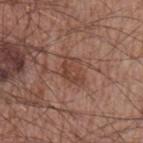Clinical impression: The lesion was photographed on a routine skin check and not biopsied; there is no pathology result. Clinical summary: A male subject aged 63 to 67. The lesion's longest dimension is about 3.5 mm. A 15 mm close-up tile from a total-body photography series done for melanoma screening. Located on the left upper arm. This is a white-light tile. The total-body-photography lesion software estimated an average lesion color of about L≈43 a*≈21 b*≈27 (CIELAB) and a normalized border contrast of about 6.5. The analysis additionally found a within-lesion color-variation index near 2.5/10 and a peripheral color-asymmetry measure near 0.5. The software also gave an automated nevus-likeness rating near 0 out of 100 and a detector confidence of about 100 out of 100 that the crop contains a lesion.